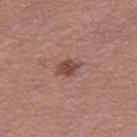Imaged during a routine full-body skin examination; the lesion was not biopsied and no histopathology is available. About 3 mm across. A female subject, aged 58 to 62. Imaged with white-light lighting. The lesion is located on the right thigh. Cropped from a total-body skin-imaging series; the visible field is about 15 mm.